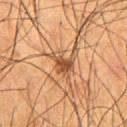Clinical impression:
This lesion was catalogued during total-body skin photography and was not selected for biopsy.
Context:
The lesion's longest dimension is about 4 mm. A lesion tile, about 15 mm wide, cut from a 3D total-body photograph. A male patient, in their mid-50s. From the abdomen. The tile uses cross-polarized illumination. An algorithmic analysis of the crop reported a lesion area of about 6.5 mm², an eccentricity of roughly 0.7, and a symmetry-axis asymmetry near 0.3. The analysis additionally found a lesion color around L≈49 a*≈21 b*≈35 in CIELAB, about 11 CIELAB-L* units darker than the surrounding skin, and a normalized lesion–skin contrast near 8. And it measured a border-irregularity index near 4.5/10 and a within-lesion color-variation index near 3.5/10. It also reported an automated nevus-likeness rating near 75 out of 100 and lesion-presence confidence of about 100/100.